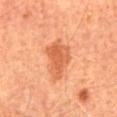Imaged during a routine full-body skin examination; the lesion was not biopsied and no histopathology is available. The subject is a male aged 63–67. The lesion is on the front of the torso. The tile uses cross-polarized illumination. An algorithmic analysis of the crop reported a lesion area of about 11 mm², an eccentricity of roughly 0.75, and two-axis asymmetry of about 0.35. The software also gave an average lesion color of about L≈55 a*≈28 b*≈38 (CIELAB), a lesion–skin lightness drop of about 10, and a lesion-to-skin contrast of about 7 (normalized; higher = more distinct). And it measured a nevus-likeness score of about 55/100 and a lesion-detection confidence of about 100/100. A 15 mm close-up extracted from a 3D total-body photography capture. About 5 mm across.